A male patient aged around 60. An algorithmic analysis of the crop reported an area of roughly 39 mm², an eccentricity of roughly 0.5, and a shape-asymmetry score of about 0.25 (0 = symmetric). The analysis additionally found an average lesion color of about L≈50 a*≈21 b*≈20 (CIELAB), roughly 10 lightness units darker than nearby skin, and a normalized border contrast of about 8. The analysis additionally found a border-irregularity rating of about 6/10 and peripheral color asymmetry of about 3.5. And it measured a nevus-likeness score of about 0/100 and a detector confidence of about 95 out of 100 that the crop contains a lesion. The lesion's longest dimension is about 7.5 mm. From the left upper arm. This is a white-light tile. A 15 mm crop from a total-body photograph taken for skin-cancer surveillance.
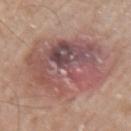<tbp_lesion>
<diagnosis>
  <histopathology>invasive melanoma arising in association with a nevus</histopathology>
  <malignancy>malignant</malignancy>
  <taxonomic_path>Malignant; Malignant melanocytic proliferations (Melanoma); Melanoma Invasive; Melanoma Invasive, Associated with a nevus</taxonomic_path>
  <breslow_mm>1.3</breslow_mm>
  <mitotic_index>3/mm²</mitotic_index>
</diagnosis>
</tbp_lesion>